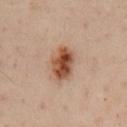The lesion was tiled from a total-body skin photograph and was not biopsied. The lesion is on the mid back. The recorded lesion diameter is about 4.5 mm. A 15 mm crop from a total-body photograph taken for skin-cancer surveillance. The tile uses cross-polarized illumination. The patient is a male approximately 55 years of age.